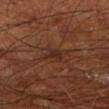<record>
  <biopsy_status>not biopsied; imaged during a skin examination</biopsy_status>
  <patient>
    <sex>male</sex>
    <age_approx>65</age_approx>
  </patient>
  <automated_metrics>
    <area_mm2_approx>4.5</area_mm2_approx>
    <eccentricity>0.7</eccentricity>
    <shape_asymmetry>0.3</shape_asymmetry>
    <cielab_L>27</cielab_L>
    <cielab_a>20</cielab_a>
    <cielab_b>25</cielab_b>
    <vs_skin_darker_L>6.0</vs_skin_darker_L>
    <vs_skin_contrast_norm>6.5</vs_skin_contrast_norm>
    <border_irregularity_0_10>4.0</border_irregularity_0_10>
    <color_variation_0_10>2.0</color_variation_0_10>
    <peripheral_color_asymmetry>1.0</peripheral_color_asymmetry>
    <nevus_likeness_0_100>0</nevus_likeness_0_100>
    <lesion_detection_confidence_0_100>90</lesion_detection_confidence_0_100>
  </automated_metrics>
  <lesion_size>
    <long_diameter_mm_approx>3.0</long_diameter_mm_approx>
  </lesion_size>
  <site>left lower leg</site>
  <lighting>cross-polarized</lighting>
  <image>
    <source>total-body photography crop</source>
    <field_of_view_mm>15</field_of_view_mm>
  </image>
</record>